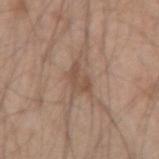  lesion_size:
    long_diameter_mm_approx: 3.0
  lighting: white-light
  site: right forearm
  patient:
    sex: male
    age_approx: 45
  automated_metrics:
    area_mm2_approx: 4.5
    eccentricity: 0.8
    shape_asymmetry: 0.4
    nevus_likeness_0_100: 0
    lesion_detection_confidence_0_100: 100
  image:
    source: total-body photography crop
    field_of_view_mm: 15